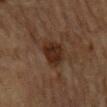image: ~15 mm tile from a whole-body skin photo
body site: the mid back
patient: male, roughly 85 years of age or older
automated metrics: a lesion area of about 10 mm², an eccentricity of roughly 0.85, and two-axis asymmetry of about 0.25; an average lesion color of about L≈23 a*≈16 b*≈23 (CIELAB), roughly 8 lightness units darker than nearby skin, and a normalized lesion–skin contrast near 9.5; a border-irregularity rating of about 2.5/10 and a color-variation rating of about 3/10; an automated nevus-likeness rating near 75 out of 100 and lesion-presence confidence of about 100/100
lighting: cross-polarized illumination